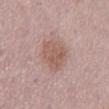Q: Is there a histopathology result?
A: catalogued during a skin exam; not biopsied
Q: What kind of image is this?
A: ~15 mm crop, total-body skin-cancer survey
Q: Where on the body is the lesion?
A: the back
Q: Patient demographics?
A: male, aged around 55
Q: How large is the lesion?
A: ~4.5 mm (longest diameter)